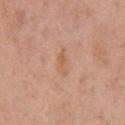The subject is a male aged 68–72. A 15 mm close-up tile from a total-body photography series done for melanoma screening. On the chest. About 3.5 mm across.